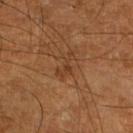| key | value |
|---|---|
| follow-up | total-body-photography surveillance lesion; no biopsy |
| TBP lesion metrics | an average lesion color of about L≈37 a*≈21 b*≈32 (CIELAB), a lesion–skin lightness drop of about 6, and a normalized lesion–skin contrast near 5.5; a border-irregularity index near 8/10; a classifier nevus-likeness of about 0/100 |
| subject | male, in their mid- to late 60s |
| image source | ~15 mm crop, total-body skin-cancer survey |
| site | the left leg |
| lesion diameter | ~3.5 mm (longest diameter) |
| lighting | cross-polarized |